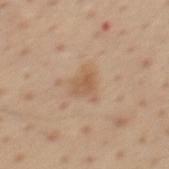{"biopsy_status": "not biopsied; imaged during a skin examination", "lesion_size": {"long_diameter_mm_approx": 3.5}, "patient": {"sex": "male", "age_approx": 55}, "automated_metrics": {"area_mm2_approx": 5.5, "eccentricity": 0.45, "shape_asymmetry": 0.4, "border_irregularity_0_10": 4.5, "color_variation_0_10": 3.0, "peripheral_color_asymmetry": 1.0, "nevus_likeness_0_100": 20, "lesion_detection_confidence_0_100": 100}, "lighting": "white-light", "site": "mid back", "image": {"source": "total-body photography crop", "field_of_view_mm": 15}}